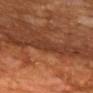Part of a total-body skin-imaging series; this lesion was reviewed on a skin check and was not flagged for biopsy.
The lesion is located on the upper back.
A lesion tile, about 15 mm wide, cut from a 3D total-body photograph.
The tile uses cross-polarized illumination.
The subject is a male roughly 60 years of age.
The lesion's longest dimension is about 5 mm.
Automated tile analysis of the lesion measured a lesion area of about 6.5 mm², a shape eccentricity near 0.95, and two-axis asymmetry of about 0.45. And it measured a lesion color around L≈33 a*≈22 b*≈29 in CIELAB, roughly 6 lightness units darker than nearby skin, and a normalized lesion–skin contrast near 5.5. And it measured a border-irregularity index near 6.5/10, internal color variation of about 1.5 on a 0–10 scale, and radial color variation of about 0.5. And it measured a nevus-likeness score of about 0/100 and a lesion-detection confidence of about 0/100.